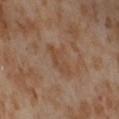Q: Is there a histopathology result?
A: catalogued during a skin exam; not biopsied
Q: Where on the body is the lesion?
A: the leg
Q: What is the imaging modality?
A: ~15 mm tile from a whole-body skin photo
Q: What did automated image analysis measure?
A: an area of roughly 7 mm², a shape eccentricity near 0.85, and a symmetry-axis asymmetry near 0.35; a border-irregularity index near 4.5/10 and a within-lesion color-variation index near 2.5/10
Q: Patient demographics?
A: female, aged 53–57
Q: How was the tile lit?
A: cross-polarized
Q: How large is the lesion?
A: about 4 mm